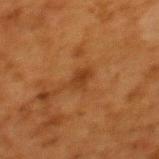<record>
<biopsy_status>not biopsied; imaged during a skin examination</biopsy_status>
<site>upper back</site>
<image>
  <source>total-body photography crop</source>
  <field_of_view_mm>15</field_of_view_mm>
</image>
<patient>
  <sex>female</sex>
  <age_approx>50</age_approx>
</patient>
<lesion_size>
  <long_diameter_mm_approx>3.0</long_diameter_mm_approx>
</lesion_size>
</record>